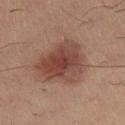The lesion was tiled from a total-body skin photograph and was not biopsied. On the right thigh. A roughly 15 mm field-of-view crop from a total-body skin photograph. The subject is a male roughly 35 years of age. The total-body-photography lesion software estimated a classifier nevus-likeness of about 95/100 and lesion-presence confidence of about 100/100. Approximately 6 mm at its widest.